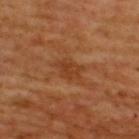| field | value |
|---|---|
| notes | total-body-photography surveillance lesion; no biopsy |
| patient | male, aged around 65 |
| illumination | cross-polarized illumination |
| image | 15 mm crop, total-body photography |
| image-analysis metrics | a lesion area of about 5.5 mm², an eccentricity of roughly 0.7, and a symmetry-axis asymmetry near 0.25; an average lesion color of about L≈38 a*≈24 b*≈35 (CIELAB) and a normalized lesion–skin contrast near 6; a classifier nevus-likeness of about 0/100 and a lesion-detection confidence of about 100/100 |
| anatomic site | the upper back |
| diameter | ~3 mm (longest diameter) |